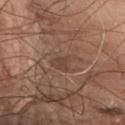| field | value |
|---|---|
| workup | total-body-photography surveillance lesion; no biopsy |
| patient | male, aged 63–67 |
| automated metrics | a mean CIELAB color near L≈43 a*≈18 b*≈25, roughly 6 lightness units darker than nearby skin, and a normalized lesion–skin contrast near 5; a border-irregularity index near 3.5/10, a color-variation rating of about 1.5/10, and peripheral color asymmetry of about 0.5; a nevus-likeness score of about 0/100 and a lesion-detection confidence of about 60/100 |
| acquisition | ~15 mm crop, total-body skin-cancer survey |
| site | the upper back |
| lesion diameter | about 3.5 mm |
| lighting | white-light illumination |